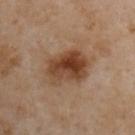Q: Was a biopsy performed?
A: no biopsy performed (imaged during a skin exam)
Q: What did automated image analysis measure?
A: a symmetry-axis asymmetry near 0.2; an average lesion color of about L≈42 a*≈20 b*≈31 (CIELAB); a nevus-likeness score of about 95/100 and lesion-presence confidence of about 100/100
Q: Patient demographics?
A: male, in their mid-50s
Q: How was this image acquired?
A: 15 mm crop, total-body photography
Q: How large is the lesion?
A: ≈5 mm
Q: What is the anatomic site?
A: the right upper arm